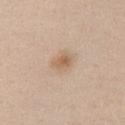Q: Was a biopsy performed?
A: no biopsy performed (imaged during a skin exam)
Q: Who is the patient?
A: female, about 40 years old
Q: How was this image acquired?
A: 15 mm crop, total-body photography
Q: Lesion size?
A: ≈2.5 mm
Q: How was the tile lit?
A: white-light
Q: What is the anatomic site?
A: the left upper arm
Q: What did automated image analysis measure?
A: a lesion area of about 4.5 mm², a shape eccentricity near 0.6, and a symmetry-axis asymmetry near 0.25; a lesion color around L≈61 a*≈17 b*≈32 in CIELAB, about 9 CIELAB-L* units darker than the surrounding skin, and a normalized lesion–skin contrast near 6.5